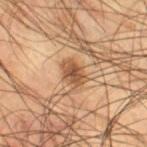Assessment:
Part of a total-body skin-imaging series; this lesion was reviewed on a skin check and was not flagged for biopsy.